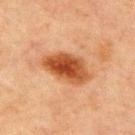- biopsy status — total-body-photography surveillance lesion; no biopsy
- site — the chest
- subject — male, in their mid-60s
- acquisition — ~15 mm crop, total-body skin-cancer survey
- tile lighting — cross-polarized
- lesion diameter — about 6 mm